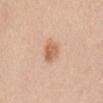Clinical impression:
This lesion was catalogued during total-body skin photography and was not selected for biopsy.